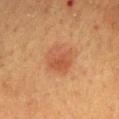{
  "image": {
    "source": "total-body photography crop",
    "field_of_view_mm": 15
  },
  "lesion_size": {
    "long_diameter_mm_approx": 3.5
  },
  "site": "mid back",
  "automated_metrics": {
    "area_mm2_approx": 9.5,
    "eccentricity": 0.4,
    "shape_asymmetry": 0.2,
    "border_irregularity_0_10": 2.0,
    "peripheral_color_asymmetry": 1.5
  },
  "lighting": "cross-polarized",
  "patient": {
    "sex": "male",
    "age_approx": 60
  }
}